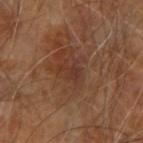follow-up — catalogued during a skin exam; not biopsied
lighting — cross-polarized illumination
patient — male, aged approximately 70
image source — total-body-photography crop, ~15 mm field of view
location — the arm